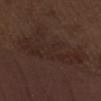Case summary:
– follow-up — no biopsy performed (imaged during a skin exam)
– site — the front of the torso
– subject — male, aged approximately 70
– lesion diameter — ~9.5 mm (longest diameter)
– lighting — white-light
– acquisition — 15 mm crop, total-body photography